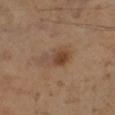Recorded during total-body skin imaging; not selected for excision or biopsy.